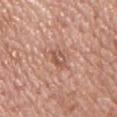Q: Lesion size?
A: ≈3 mm
Q: What is the imaging modality?
A: ~15 mm crop, total-body skin-cancer survey
Q: Patient demographics?
A: male, roughly 75 years of age
Q: Lesion location?
A: the front of the torso
Q: How was the tile lit?
A: white-light illumination